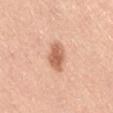follow-up = no biopsy performed (imaged during a skin exam)
automated lesion analysis = a border-irregularity index near 2.5/10 and peripheral color asymmetry of about 1
anatomic site = the right thigh
imaging modality = ~15 mm crop, total-body skin-cancer survey
patient = male, approximately 50 years of age